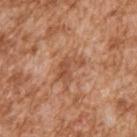Clinical impression: Recorded during total-body skin imaging; not selected for excision or biopsy. Clinical summary: The lesion's longest dimension is about 3.5 mm. A lesion tile, about 15 mm wide, cut from a 3D total-body photograph. The lesion is on the right upper arm. This is a white-light tile. A male patient, aged around 45.